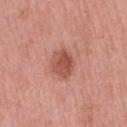The lesion was tiled from a total-body skin photograph and was not biopsied.
The subject is a female in their 70s.
Measured at roughly 3.5 mm in maximum diameter.
A 15 mm close-up tile from a total-body photography series done for melanoma screening.
Automated image analysis of the tile measured a mean CIELAB color near L≈52 a*≈27 b*≈29 and a lesion-to-skin contrast of about 7.5 (normalized; higher = more distinct). The analysis additionally found a border-irregularity index near 1.5/10 and a peripheral color-asymmetry measure near 1. The software also gave a classifier nevus-likeness of about 80/100 and lesion-presence confidence of about 100/100.
This is a white-light tile.
The lesion is on the lower back.